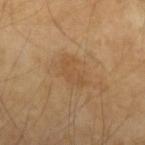Notes:
* notes: total-body-photography surveillance lesion; no biopsy
* body site: the left forearm
* patient: male, about 50 years old
* lighting: cross-polarized illumination
* image: total-body-photography crop, ~15 mm field of view